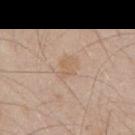Q: Was a biopsy performed?
A: imaged on a skin check; not biopsied
Q: What kind of image is this?
A: ~15 mm tile from a whole-body skin photo
Q: Patient demographics?
A: male, in their 50s
Q: Where on the body is the lesion?
A: the mid back
Q: How was the tile lit?
A: white-light illumination
Q: How large is the lesion?
A: about 2.5 mm